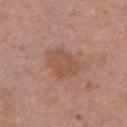Assessment:
Imaged during a routine full-body skin examination; the lesion was not biopsied and no histopathology is available.
Clinical summary:
A female subject, aged around 40. The recorded lesion diameter is about 4 mm. Imaged with white-light lighting. The lesion is located on the chest. A 15 mm crop from a total-body photograph taken for skin-cancer surveillance.